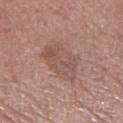{"lighting": "white-light", "patient": {"sex": "female", "age_approx": 75}, "lesion_size": {"long_diameter_mm_approx": 5.5}, "site": "left lower leg", "image": {"source": "total-body photography crop", "field_of_view_mm": 15}}